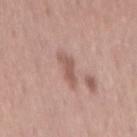workup: catalogued during a skin exam; not biopsied | image: total-body-photography crop, ~15 mm field of view | lesion diameter: about 4 mm | location: the back | patient: female, about 55 years old.